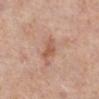| field | value |
|---|---|
| location | the right lower leg |
| tile lighting | white-light illumination |
| subject | female, aged approximately 65 |
| imaging modality | total-body-photography crop, ~15 mm field of view |